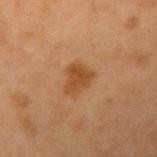No biopsy was performed on this lesion — it was imaged during a full skin examination and was not determined to be concerning. Imaged with cross-polarized lighting. A female subject, approximately 40 years of age. Automated tile analysis of the lesion measured a lesion color around L≈40 a*≈21 b*≈34 in CIELAB, about 8 CIELAB-L* units darker than the surrounding skin, and a normalized lesion–skin contrast near 8. The analysis additionally found a border-irregularity index near 4/10 and radial color variation of about 0.5. The analysis additionally found a nevus-likeness score of about 75/100 and a detector confidence of about 100 out of 100 that the crop contains a lesion. The lesion is located on the right upper arm. Cropped from a whole-body photographic skin survey; the tile spans about 15 mm. Approximately 3 mm at its widest.